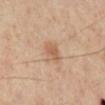{
  "biopsy_status": "not biopsied; imaged during a skin examination",
  "site": "right lower leg",
  "lesion_size": {
    "long_diameter_mm_approx": 2.5
  },
  "patient": {
    "sex": "male",
    "age_approx": 55
  },
  "automated_metrics": {
    "cielab_L": 58,
    "cielab_a": 21,
    "cielab_b": 33,
    "vs_skin_darker_L": 9.0,
    "vs_skin_contrast_norm": 6.0,
    "nevus_likeness_0_100": 65
  },
  "image": {
    "source": "total-body photography crop",
    "field_of_view_mm": 15
  }
}